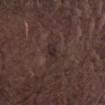workup: no biopsy performed (imaged during a skin exam)
patient: male, approximately 40 years of age
anatomic site: the right thigh
image source: 15 mm crop, total-body photography
illumination: white-light